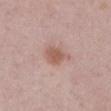– follow-up: catalogued during a skin exam; not biopsied
– subject: male, aged around 50
– body site: the arm
– lighting: white-light
– imaging modality: ~15 mm tile from a whole-body skin photo
– lesion size: about 3.5 mm
– TBP lesion metrics: an average lesion color of about L≈57 a*≈20 b*≈26 (CIELAB) and a normalized lesion–skin contrast near 7